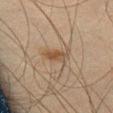The lesion was photographed on a routine skin check and not biopsied; there is no pathology result. On the left thigh. Measured at roughly 3.5 mm in maximum diameter. A region of skin cropped from a whole-body photographic capture, roughly 15 mm wide. A male patient in their mid-40s. Captured under cross-polarized illumination.